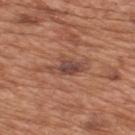follow-up: imaged on a skin check; not biopsied
body site: the upper back
subject: male, aged 63 to 67
illumination: white-light illumination
imaging modality: ~15 mm tile from a whole-body skin photo
lesion diameter: ~4 mm (longest diameter)